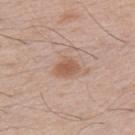- workup — imaged on a skin check; not biopsied
- illumination — white-light illumination
- automated metrics — a classifier nevus-likeness of about 80/100 and a detector confidence of about 100 out of 100 that the crop contains a lesion
- anatomic site — the left upper arm
- subject — male, aged 48 to 52
- image source — 15 mm crop, total-body photography